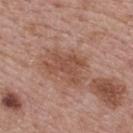Q: Was a biopsy performed?
A: no biopsy performed (imaged during a skin exam)
Q: Illumination type?
A: white-light illumination
Q: Patient demographics?
A: female, aged around 65
Q: What did automated image analysis measure?
A: an average lesion color of about L≈49 a*≈22 b*≈29 (CIELAB), about 9 CIELAB-L* units darker than the surrounding skin, and a normalized lesion–skin contrast near 6.5
Q: How large is the lesion?
A: about 4.5 mm
Q: What is the imaging modality?
A: 15 mm crop, total-body photography
Q: What is the anatomic site?
A: the upper back